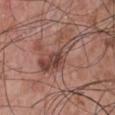<record>
  <biopsy_status>not biopsied; imaged during a skin examination</biopsy_status>
  <lesion_size>
    <long_diameter_mm_approx>7.0</long_diameter_mm_approx>
  </lesion_size>
  <patient>
    <sex>male</sex>
    <age_approx>70</age_approx>
  </patient>
  <site>chest</site>
  <image>
    <source>total-body photography crop</source>
    <field_of_view_mm>15</field_of_view_mm>
  </image>
</record>